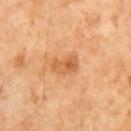Acquisition and patient details: A 15 mm close-up extracted from a 3D total-body photography capture. A male subject, in their mid- to late 60s. Longest diameter approximately 3 mm.